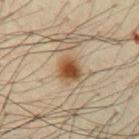{"biopsy_status": "not biopsied; imaged during a skin examination", "site": "chest", "lighting": "cross-polarized", "lesion_size": {"long_diameter_mm_approx": 3.0}, "automated_metrics": {"color_variation_0_10": 5.0, "peripheral_color_asymmetry": 1.5, "nevus_likeness_0_100": 95}, "image": {"source": "total-body photography crop", "field_of_view_mm": 15}, "patient": {"sex": "male", "age_approx": 50}}